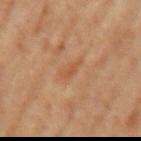The lesion was photographed on a routine skin check and not biopsied; there is no pathology result.
The recorded lesion diameter is about 3 mm.
A female subject, in their mid-60s.
On the left upper arm.
The tile uses cross-polarized illumination.
This image is a 15 mm lesion crop taken from a total-body photograph.